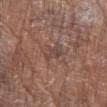biopsy_status: not biopsied; imaged during a skin examination
lighting: white-light
patient:
  sex: female
  age_approx: 75
image:
  source: total-body photography crop
  field_of_view_mm: 15
site: right lower leg